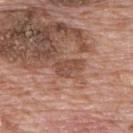Imaged during a routine full-body skin examination; the lesion was not biopsied and no histopathology is available.
Cropped from a whole-body photographic skin survey; the tile spans about 15 mm.
Automated tile analysis of the lesion measured a mean CIELAB color near L≈48 a*≈21 b*≈28, roughly 8 lightness units darker than nearby skin, and a lesion-to-skin contrast of about 6 (normalized; higher = more distinct). The software also gave a nevus-likeness score of about 0/100.
Captured under white-light illumination.
A male subject aged approximately 70.
The lesion is on the upper back.